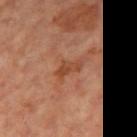No biopsy was performed on this lesion — it was imaged during a full skin examination and was not determined to be concerning.
A female patient in their mid-60s.
The lesion-visualizer software estimated an outline eccentricity of about 0.9 (0 = round, 1 = elongated) and a shape-asymmetry score of about 0.45 (0 = symmetric). The software also gave a lesion color around L≈45 a*≈25 b*≈33 in CIELAB, a lesion–skin lightness drop of about 8, and a lesion-to-skin contrast of about 7 (normalized; higher = more distinct). And it measured a border-irregularity rating of about 5/10, internal color variation of about 2.5 on a 0–10 scale, and peripheral color asymmetry of about 1.
About 3.5 mm across.
Imaged with cross-polarized lighting.
This image is a 15 mm lesion crop taken from a total-body photograph.
The lesion is located on the right thigh.